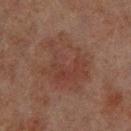<record>
<biopsy_status>not biopsied; imaged during a skin examination</biopsy_status>
<lighting>cross-polarized</lighting>
<lesion_size>
  <long_diameter_mm_approx>7.0</long_diameter_mm_approx>
</lesion_size>
<image>
  <source>total-body photography crop</source>
  <field_of_view_mm>15</field_of_view_mm>
</image>
<site>right lower leg</site>
<patient>
  <sex>male</sex>
  <age_approx>70</age_approx>
</patient>
</record>